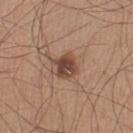Q: Was this lesion biopsied?
A: imaged on a skin check; not biopsied
Q: What kind of image is this?
A: ~15 mm tile from a whole-body skin photo
Q: What is the anatomic site?
A: the leg
Q: What is the lesion's diameter?
A: ≈3 mm
Q: What lighting was used for the tile?
A: white-light
Q: Automated lesion metrics?
A: a lesion color around L≈45 a*≈19 b*≈26 in CIELAB and about 12 CIELAB-L* units darker than the surrounding skin
Q: What are the patient's age and sex?
A: male, aged around 30